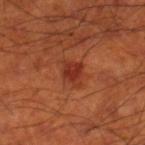notes: no biopsy performed (imaged during a skin exam)
patient: male, roughly 70 years of age
diameter: ≈3 mm
lighting: cross-polarized illumination
site: the right thigh
acquisition: 15 mm crop, total-body photography